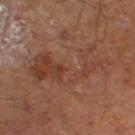{"lighting": "cross-polarized", "site": "leg", "lesion_size": {"long_diameter_mm_approx": 9.0}, "patient": {"sex": "male", "age_approx": 70}, "automated_metrics": {"area_mm2_approx": 26.0, "eccentricity": 0.9, "shape_asymmetry": 0.55, "cielab_L": 32, "cielab_a": 17, "cielab_b": 23, "vs_skin_darker_L": 5.0, "vs_skin_contrast_norm": 5.0, "nevus_likeness_0_100": 0, "lesion_detection_confidence_0_100": 100}, "image": {"source": "total-body photography crop", "field_of_view_mm": 15}}